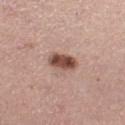workup=total-body-photography surveillance lesion; no biopsy
subject=female, about 40 years old
location=the leg
acquisition=15 mm crop, total-body photography